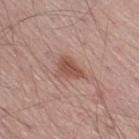| key | value |
|---|---|
| workup | no biopsy performed (imaged during a skin exam) |
| diameter | ≈3.5 mm |
| patient | male, about 55 years old |
| acquisition | ~15 mm tile from a whole-body skin photo |
| TBP lesion metrics | an eccentricity of roughly 0.65 and a symmetry-axis asymmetry near 0.45; roughly 10 lightness units darker than nearby skin and a lesion-to-skin contrast of about 7.5 (normalized; higher = more distinct); border irregularity of about 4.5 on a 0–10 scale, a within-lesion color-variation index near 2.5/10, and peripheral color asymmetry of about 1 |
| location | the right thigh |